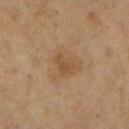biopsy_status: not biopsied; imaged during a skin examination